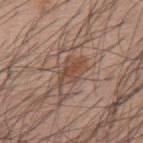Part of a total-body skin-imaging series; this lesion was reviewed on a skin check and was not flagged for biopsy.